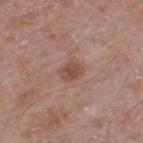Q: Was this lesion biopsied?
A: no biopsy performed (imaged during a skin exam)
Q: Patient demographics?
A: male, aged 58 to 62
Q: Automated lesion metrics?
A: an outline eccentricity of about 0.6 (0 = round, 1 = elongated); border irregularity of about 2 on a 0–10 scale, a color-variation rating of about 3/10, and a peripheral color-asymmetry measure near 1
Q: Illumination type?
A: white-light
Q: Lesion size?
A: ≈2.5 mm
Q: How was this image acquired?
A: ~15 mm crop, total-body skin-cancer survey
Q: What is the anatomic site?
A: the right thigh